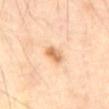On the abdomen. A lesion tile, about 15 mm wide, cut from a 3D total-body photograph. The tile uses cross-polarized illumination. Automated image analysis of the tile measured a lesion color around L≈68 a*≈22 b*≈39 in CIELAB, a lesion–skin lightness drop of about 13, and a lesion-to-skin contrast of about 8 (normalized; higher = more distinct). The analysis additionally found border irregularity of about 1.5 on a 0–10 scale, a within-lesion color-variation index near 4.5/10, and a peripheral color-asymmetry measure near 1.5. Longest diameter approximately 2.5 mm. The patient is a male approximately 70 years of age.